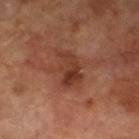Context: Automated tile analysis of the lesion measured a footprint of about 9 mm², an eccentricity of roughly 0.75, and a symmetry-axis asymmetry near 0.4. And it measured an automated nevus-likeness rating near 5 out of 100 and a lesion-detection confidence of about 100/100. The lesion is located on the left lower leg. The subject is a male aged around 70. The tile uses cross-polarized illumination. A region of skin cropped from a whole-body photographic capture, roughly 15 mm wide. Longest diameter approximately 4 mm.